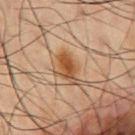• follow-up: total-body-photography surveillance lesion; no biopsy